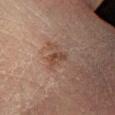<lesion>
  <biopsy_status>not biopsied; imaged during a skin examination</biopsy_status>
  <site>left lower leg</site>
  <lighting>cross-polarized</lighting>
  <patient>
    <sex>female</sex>
    <age_approx>55</age_approx>
  </patient>
  <lesion_size>
    <long_diameter_mm_approx>2.5</long_diameter_mm_approx>
  </lesion_size>
  <image>
    <source>total-body photography crop</source>
    <field_of_view_mm>15</field_of_view_mm>
  </image>
</lesion>